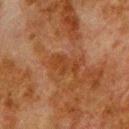<tbp_lesion>
<biopsy_status>not biopsied; imaged during a skin examination</biopsy_status>
<lighting>cross-polarized</lighting>
<lesion_size>
  <long_diameter_mm_approx>4.0</long_diameter_mm_approx>
</lesion_size>
<site>upper back</site>
<patient>
  <sex>male</sex>
  <age_approx>80</age_approx>
</patient>
<image>
  <source>total-body photography crop</source>
  <field_of_view_mm>15</field_of_view_mm>
</image>
<automated_metrics>
  <area_mm2_approx>5.5</area_mm2_approx>
  <eccentricity>0.9</eccentricity>
  <shape_asymmetry>0.3</shape_asymmetry>
  <cielab_L>32</cielab_L>
  <cielab_a>21</cielab_a>
  <cielab_b>31</cielab_b>
  <vs_skin_darker_L>5.0</vs_skin_darker_L>
  <vs_skin_contrast_norm>5.5</vs_skin_contrast_norm>
</automated_metrics>
</tbp_lesion>